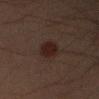notes — no biopsy performed (imaged during a skin exam) | anatomic site — the right upper arm | illumination — cross-polarized illumination | subject — male, aged 43 to 47 | automated metrics — a lesion color around L≈16 a*≈14 b*≈16 in CIELAB and roughly 6 lightness units darker than nearby skin; a peripheral color-asymmetry measure near 1; a nevus-likeness score of about 100/100 and a detector confidence of about 100 out of 100 that the crop contains a lesion | image source — ~15 mm crop, total-body skin-cancer survey | lesion size — ~3.5 mm (longest diameter).